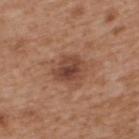Image and clinical context:
A lesion tile, about 15 mm wide, cut from a 3D total-body photograph. Measured at roughly 3.5 mm in maximum diameter. A male subject, aged approximately 65. The tile uses white-light illumination. Located on the back.